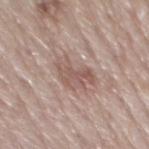Case summary:
– image: 15 mm crop, total-body photography
– patient: male, in their mid- to late 70s
– location: the back